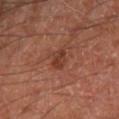Imaged during a routine full-body skin examination; the lesion was not biopsied and no histopathology is available. A male subject, aged 68 to 72. A 15 mm close-up tile from a total-body photography series done for melanoma screening. Located on the leg.